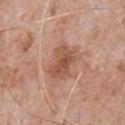Case summary:
* biopsy status: total-body-photography surveillance lesion; no biopsy
* diameter: about 4.5 mm
* patient: male, aged around 65
* image-analysis metrics: an area of roughly 9.5 mm² and a shape-asymmetry score of about 0.2 (0 = symmetric); lesion-presence confidence of about 100/100
* body site: the chest
* acquisition: ~15 mm tile from a whole-body skin photo
* lighting: white-light illumination